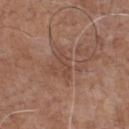notes: total-body-photography surveillance lesion; no biopsy | automated lesion analysis: border irregularity of about 5 on a 0–10 scale, a within-lesion color-variation index near 0/10, and peripheral color asymmetry of about 0; a nevus-likeness score of about 0/100 and a detector confidence of about 85 out of 100 that the crop contains a lesion | lesion size: ≈3 mm | patient: male, aged approximately 70 | illumination: white-light illumination | body site: the chest | imaging modality: 15 mm crop, total-body photography.